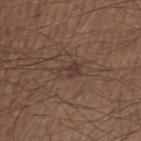No biopsy was performed on this lesion — it was imaged during a full skin examination and was not determined to be concerning. A male patient, aged approximately 50. A region of skin cropped from a whole-body photographic capture, roughly 15 mm wide. Measured at roughly 2.5 mm in maximum diameter. Located on the leg.